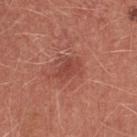follow-up: total-body-photography surveillance lesion; no biopsy